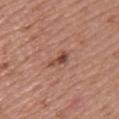Clinical impression:
Imaged during a routine full-body skin examination; the lesion was not biopsied and no histopathology is available.
Context:
From the left upper arm. A female subject, about 50 years old. This image is a 15 mm lesion crop taken from a total-body photograph. This is a white-light tile. The lesion-visualizer software estimated an area of roughly 3.5 mm², a shape eccentricity near 0.9, and a shape-asymmetry score of about 0.45 (0 = symmetric). The analysis additionally found roughly 11 lightness units darker than nearby skin. The software also gave a border-irregularity index near 5/10, a within-lesion color-variation index near 2/10, and peripheral color asymmetry of about 0.5. The software also gave a classifier nevus-likeness of about 30/100 and a detector confidence of about 100 out of 100 that the crop contains a lesion.